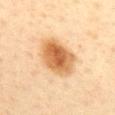| feature | finding |
|---|---|
| notes | total-body-photography surveillance lesion; no biopsy |
| patient | female, aged 43 to 47 |
| lesion diameter | ~5.5 mm (longest diameter) |
| imaging modality | total-body-photography crop, ~15 mm field of view |
| site | the mid back |